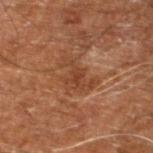follow-up: imaged on a skin check; not biopsied | subject: male, roughly 60 years of age | image: 15 mm crop, total-body photography | size: ~3 mm (longest diameter) | lighting: cross-polarized | anatomic site: the right leg.